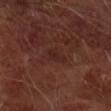follow-up: catalogued during a skin exam; not biopsied | diameter: ~2.5 mm (longest diameter) | TBP lesion metrics: an outline eccentricity of about 0.8 (0 = round, 1 = elongated) and two-axis asymmetry of about 0.4; roughly 4 lightness units darker than nearby skin and a normalized lesion–skin contrast near 5; a border-irregularity rating of about 4/10 and a color-variation rating of about 0.5/10 | image: 15 mm crop, total-body photography | lighting: cross-polarized | subject: male, in their mid- to late 60s | body site: the right forearm.